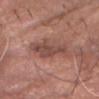{
  "biopsy_status": "not biopsied; imaged during a skin examination"
}